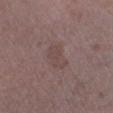Clinical impression: Imaged during a routine full-body skin examination; the lesion was not biopsied and no histopathology is available.